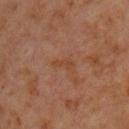Captured under cross-polarized illumination. The patient is a male in their 60s. A 15 mm close-up extracted from a 3D total-body photography capture. The lesion is on the chest.